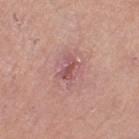Assessment: Imaged during a routine full-body skin examination; the lesion was not biopsied and no histopathology is available.